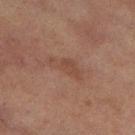Clinical impression:
No biopsy was performed on this lesion — it was imaged during a full skin examination and was not determined to be concerning.
Context:
The lesion is on the left thigh. This image is a 15 mm lesion crop taken from a total-body photograph. The lesion-visualizer software estimated an area of roughly 5.5 mm², an eccentricity of roughly 0.95, and two-axis asymmetry of about 0.4. It also reported a lesion color around L≈39 a*≈18 b*≈24 in CIELAB and a lesion–skin lightness drop of about 5. It also reported internal color variation of about 1.5 on a 0–10 scale and a peripheral color-asymmetry measure near 0.5. The software also gave an automated nevus-likeness rating near 0 out of 100 and a lesion-detection confidence of about 100/100. A female subject aged 58–62.